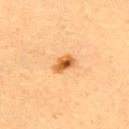A lesion tile, about 15 mm wide, cut from a 3D total-body photograph.
The subject is a female aged 28 to 32.
The total-body-photography lesion software estimated about 15 CIELAB-L* units darker than the surrounding skin and a lesion-to-skin contrast of about 9.5 (normalized; higher = more distinct). The software also gave border irregularity of about 2.5 on a 0–10 scale, a color-variation rating of about 8/10, and peripheral color asymmetry of about 2.5. The analysis additionally found an automated nevus-likeness rating near 95 out of 100 and lesion-presence confidence of about 100/100.
This is a cross-polarized tile.
The lesion is on the upper back.
Measured at roughly 3 mm in maximum diameter.